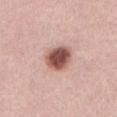No biopsy was performed on this lesion — it was imaged during a full skin examination and was not determined to be concerning.
A 15 mm close-up tile from a total-body photography series done for melanoma screening.
The tile uses white-light illumination.
A female patient approximately 70 years of age.
Longest diameter approximately 4 mm.
From the abdomen.